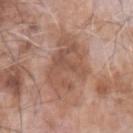The lesion was photographed on a routine skin check and not biopsied; there is no pathology result. A male patient approximately 75 years of age. A 15 mm close-up extracted from a 3D total-body photography capture. The total-body-photography lesion software estimated internal color variation of about 4 on a 0–10 scale and radial color variation of about 1.5. Located on the left forearm. The recorded lesion diameter is about 7.5 mm. Captured under white-light illumination.